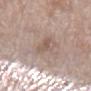Q: What did automated image analysis measure?
A: a mean CIELAB color near L≈55 a*≈16 b*≈25, a lesion–skin lightness drop of about 8, and a lesion-to-skin contrast of about 6 (normalized; higher = more distinct); a border-irregularity index near 4.5/10, internal color variation of about 0 on a 0–10 scale, and radial color variation of about 0; a classifier nevus-likeness of about 0/100
Q: What lighting was used for the tile?
A: white-light illumination
Q: Who is the patient?
A: female, aged around 75
Q: Lesion size?
A: ~3.5 mm (longest diameter)
Q: What is the anatomic site?
A: the left lower leg
Q: How was this image acquired?
A: 15 mm crop, total-body photography